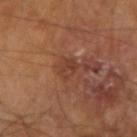Imaged during a routine full-body skin examination; the lesion was not biopsied and no histopathology is available.
The lesion is on the arm.
Cropped from a total-body skin-imaging series; the visible field is about 15 mm.
Longest diameter approximately 3 mm.
A male patient aged around 65.
Captured under cross-polarized illumination.